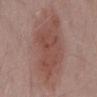  biopsy_status: not biopsied; imaged during a skin examination
  lesion_size:
    long_diameter_mm_approx: 10.0
  image:
    source: total-body photography crop
    field_of_view_mm: 15
  site: mid back
  patient:
    sex: male
    age_approx: 55